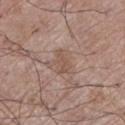The lesion was tiled from a total-body skin photograph and was not biopsied. The lesion-visualizer software estimated a lesion area of about 3.5 mm², an outline eccentricity of about 0.9 (0 = round, 1 = elongated), and a shape-asymmetry score of about 0.4 (0 = symmetric). It also reported a border-irregularity index near 4/10 and peripheral color asymmetry of about 0.5. A male patient aged around 70. Captured under white-light illumination. A 15 mm crop from a total-body photograph taken for skin-cancer surveillance. Located on the leg. Longest diameter approximately 3 mm.